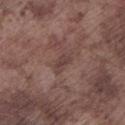Assessment: The lesion was photographed on a routine skin check and not biopsied; there is no pathology result. Background: The lesion is located on the left lower leg. Measured at roughly 3 mm in maximum diameter. The tile uses white-light illumination. Cropped from a total-body skin-imaging series; the visible field is about 15 mm. The patient is a male aged approximately 75.